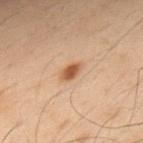| field | value |
|---|---|
| follow-up | total-body-photography surveillance lesion; no biopsy |
| automated lesion analysis | a classifier nevus-likeness of about 100/100 and lesion-presence confidence of about 100/100 |
| image | ~15 mm tile from a whole-body skin photo |
| subject | male, in their 50s |
| lesion size | ≈2.5 mm |
| body site | the upper back |